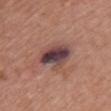Clinical summary: A 15 mm close-up tile from a total-body photography series done for melanoma screening. Longest diameter approximately 4.5 mm. A female patient, about 65 years old. The total-body-photography lesion software estimated an average lesion color of about L≈41 a*≈20 b*≈17 (CIELAB), about 15 CIELAB-L* units darker than the surrounding skin, and a lesion-to-skin contrast of about 13.5 (normalized; higher = more distinct). The analysis additionally found a border-irregularity rating of about 2.5/10, a color-variation rating of about 8.5/10, and a peripheral color-asymmetry measure near 2.5. It also reported a classifier nevus-likeness of about 0/100 and lesion-presence confidence of about 95/100. The lesion is on the front of the torso. Captured under white-light illumination.